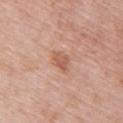Q: Was a biopsy performed?
A: imaged on a skin check; not biopsied
Q: Who is the patient?
A: female, approximately 50 years of age
Q: What is the anatomic site?
A: the upper back
Q: Lesion size?
A: ≈3 mm
Q: What is the imaging modality?
A: ~15 mm tile from a whole-body skin photo
Q: How was the tile lit?
A: white-light illumination